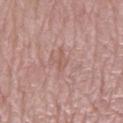patient = female, about 65 years old | lighting = white-light | image source = 15 mm crop, total-body photography | location = the left lower leg | diameter = about 3 mm.